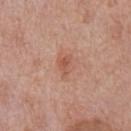Impression:
Part of a total-body skin-imaging series; this lesion was reviewed on a skin check and was not flagged for biopsy.
Background:
Automated tile analysis of the lesion measured a footprint of about 3.5 mm², an outline eccentricity of about 0.8 (0 = round, 1 = elongated), and a shape-asymmetry score of about 0.45 (0 = symmetric). The analysis additionally found a lesion-to-skin contrast of about 6 (normalized; higher = more distinct). The software also gave a border-irregularity rating of about 4/10 and a within-lesion color-variation index near 2/10. A male patient in their 70s. This image is a 15 mm lesion crop taken from a total-body photograph. This is a white-light tile. The lesion is on the chest. The recorded lesion diameter is about 2.5 mm.